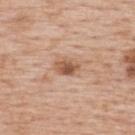Imaged during a routine full-body skin examination; the lesion was not biopsied and no histopathology is available.
Imaged with white-light lighting.
Located on the upper back.
Automated image analysis of the tile measured a mean CIELAB color near L≈56 a*≈21 b*≈32. And it measured border irregularity of about 2 on a 0–10 scale, internal color variation of about 4.5 on a 0–10 scale, and a peripheral color-asymmetry measure near 1.5.
A close-up tile cropped from a whole-body skin photograph, about 15 mm across.
The recorded lesion diameter is about 3 mm.
A female patient approximately 65 years of age.